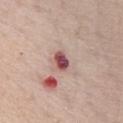The lesion was photographed on a routine skin check and not biopsied; there is no pathology result. A female subject, roughly 65 years of age. A 15 mm crop from a total-body photograph taken for skin-cancer surveillance. On the front of the torso. Longest diameter approximately 2.5 mm. The tile uses white-light illumination.